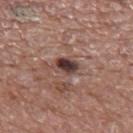Assessment: Imaged during a routine full-body skin examination; the lesion was not biopsied and no histopathology is available. Context: Cropped from a whole-body photographic skin survey; the tile spans about 15 mm. Longest diameter approximately 3 mm. The patient is a male in their 70s. An algorithmic analysis of the crop reported a lesion color around L≈39 a*≈18 b*≈20 in CIELAB, roughly 15 lightness units darker than nearby skin, and a normalized border contrast of about 12. From the upper back. Captured under white-light illumination.